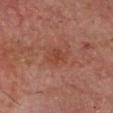The subject is a male about 65 years old.
Measured at roughly 3 mm in maximum diameter.
Imaged with cross-polarized lighting.
On the head or neck.
A lesion tile, about 15 mm wide, cut from a 3D total-body photograph.